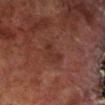The lesion was tiled from a total-body skin photograph and was not biopsied.
The subject is a male approximately 70 years of age.
The total-body-photography lesion software estimated a lesion-detection confidence of about 100/100.
This is a cross-polarized tile.
The lesion is on the right lower leg.
A roughly 15 mm field-of-view crop from a total-body skin photograph.
About 3 mm across.